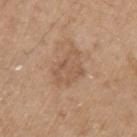Part of a total-body skin-imaging series; this lesion was reviewed on a skin check and was not flagged for biopsy.
A male patient aged approximately 70.
This image is a 15 mm lesion crop taken from a total-body photograph.
The lesion is on the left upper arm.
Measured at roughly 5 mm in maximum diameter.